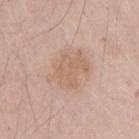Q: Is there a histopathology result?
A: total-body-photography surveillance lesion; no biopsy
Q: What are the patient's age and sex?
A: male, aged around 25
Q: How was this image acquired?
A: ~15 mm crop, total-body skin-cancer survey
Q: Lesion location?
A: the arm